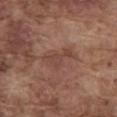Clinical impression: Captured during whole-body skin photography for melanoma surveillance; the lesion was not biopsied. Clinical summary: Captured under white-light illumination. Cropped from a total-body skin-imaging series; the visible field is about 15 mm. The total-body-photography lesion software estimated an area of roughly 5.5 mm², an outline eccentricity of about 0.85 (0 = round, 1 = elongated), and a shape-asymmetry score of about 0.6 (0 = symmetric). The analysis additionally found a border-irregularity index near 6.5/10, internal color variation of about 1.5 on a 0–10 scale, and peripheral color asymmetry of about 0.5. The analysis additionally found a classifier nevus-likeness of about 0/100 and a detector confidence of about 100 out of 100 that the crop contains a lesion. A male patient approximately 75 years of age. From the abdomen. Approximately 4 mm at its widest.